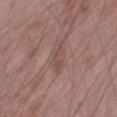Case summary:
– notes — catalogued during a skin exam; not biopsied
– acquisition — total-body-photography crop, ~15 mm field of view
– patient — male, about 50 years old
– illumination — white-light
– anatomic site — the right thigh
– image-analysis metrics — a footprint of about 4 mm², an eccentricity of roughly 0.95, and two-axis asymmetry of about 0.35; a border-irregularity index near 4.5/10, a within-lesion color-variation index near 0.5/10, and peripheral color asymmetry of about 0; an automated nevus-likeness rating near 0 out of 100